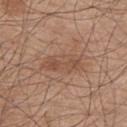| key | value |
|---|---|
| biopsy status | no biopsy performed (imaged during a skin exam) |
| patient | male, in their mid-40s |
| lesion diameter | ≈5.5 mm |
| lighting | white-light illumination |
| site | the upper back |
| automated lesion analysis | a border-irregularity rating of about 3/10 and peripheral color asymmetry of about 1 |
| image | ~15 mm tile from a whole-body skin photo |